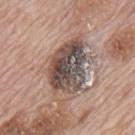Clinical impression:
Recorded during total-body skin imaging; not selected for excision or biopsy.
Context:
An algorithmic analysis of the crop reported a normalized lesion–skin contrast near 12. The software also gave internal color variation of about 10 on a 0–10 scale and radial color variation of about 3. A male patient in their 70s. A 15 mm crop from a total-body photograph taken for skin-cancer surveillance. From the mid back. Captured under white-light illumination. Longest diameter approximately 6.5 mm.